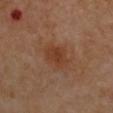follow-up: imaged on a skin check; not biopsied
subject: female, roughly 55 years of age
lighting: cross-polarized illumination
automated metrics: lesion-presence confidence of about 100/100
image source: 15 mm crop, total-body photography
lesion diameter: about 3 mm
site: the front of the torso